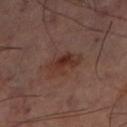Assessment:
Part of a total-body skin-imaging series; this lesion was reviewed on a skin check and was not flagged for biopsy.
Background:
A 15 mm close-up extracted from a 3D total-body photography capture. Located on the left thigh. The tile uses cross-polarized illumination.